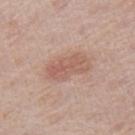Impression: Captured during whole-body skin photography for melanoma surveillance; the lesion was not biopsied. Context: A female subject, aged 58–62. Automated tile analysis of the lesion measured border irregularity of about 2 on a 0–10 scale, internal color variation of about 4 on a 0–10 scale, and a peripheral color-asymmetry measure near 1.5. The software also gave a nevus-likeness score of about 50/100 and lesion-presence confidence of about 100/100. A 15 mm close-up tile from a total-body photography series done for melanoma screening. The recorded lesion diameter is about 5 mm. On the right thigh.